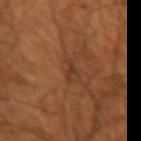{
  "lesion_size": {
    "long_diameter_mm_approx": 2.5
  },
  "patient": {
    "sex": "male",
    "age_approx": 50
  },
  "site": "left upper arm",
  "image": {
    "source": "total-body photography crop",
    "field_of_view_mm": 15
  }
}